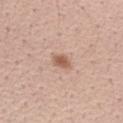workup=total-body-photography surveillance lesion; no biopsy | patient=female, aged approximately 40 | automated lesion analysis=an average lesion color of about L≈58 a*≈21 b*≈29 (CIELAB) and roughly 11 lightness units darker than nearby skin; a color-variation rating of about 2/10 and a peripheral color-asymmetry measure near 0.5; an automated nevus-likeness rating near 90 out of 100 and a detector confidence of about 100 out of 100 that the crop contains a lesion | anatomic site=the right forearm | illumination=white-light illumination | acquisition=~15 mm crop, total-body skin-cancer survey | size=~2.5 mm (longest diameter).